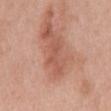No biopsy was performed on this lesion — it was imaged during a full skin examination and was not determined to be concerning. A roughly 15 mm field-of-view crop from a total-body skin photograph. The tile uses white-light illumination. The lesion's longest dimension is about 5 mm. The subject is a female roughly 40 years of age. The lesion-visualizer software estimated a lesion–skin lightness drop of about 9 and a lesion-to-skin contrast of about 6 (normalized; higher = more distinct). The analysis additionally found a border-irregularity rating of about 7.5/10 and a peripheral color-asymmetry measure near 0. The lesion is on the mid back.